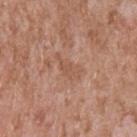<lesion>
<biopsy_status>not biopsied; imaged during a skin examination</biopsy_status>
<automated_metrics>
  <eccentricity>0.85</eccentricity>
  <shape_asymmetry>0.4</shape_asymmetry>
  <vs_skin_darker_L>6.0</vs_skin_darker_L>
  <nevus_likeness_0_100>0</nevus_likeness_0_100>
  <lesion_detection_confidence_0_100>100</lesion_detection_confidence_0_100>
</automated_metrics>
<site>left upper arm</site>
<image>
  <source>total-body photography crop</source>
  <field_of_view_mm>15</field_of_view_mm>
</image>
<lesion_size>
  <long_diameter_mm_approx>3.5</long_diameter_mm_approx>
</lesion_size>
<lighting>white-light</lighting>
<patient>
  <sex>male</sex>
  <age_approx>45</age_approx>
</patient>
</lesion>